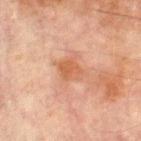Clinical summary: The lesion is located on the left lower leg. This is a cross-polarized tile. The total-body-photography lesion software estimated an area of roughly 5.5 mm² and a symmetry-axis asymmetry near 0.25. The analysis additionally found a border-irregularity rating of about 2.5/10 and radial color variation of about 1. The analysis additionally found lesion-presence confidence of about 100/100. Approximately 3 mm at its widest. The subject is a male roughly 70 years of age. A roughly 15 mm field-of-view crop from a total-body skin photograph.